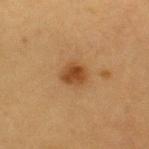workup: imaged on a skin check; not biopsied | lighting: cross-polarized illumination | patient: male, about 85 years old or older | lesion diameter: ~3 mm (longest diameter) | image source: ~15 mm crop, total-body skin-cancer survey | image-analysis metrics: a lesion area of about 4.5 mm² and a symmetry-axis asymmetry near 0.2; a border-irregularity index near 2/10, a within-lesion color-variation index near 2.5/10, and a peripheral color-asymmetry measure near 1 | body site: the right upper arm.